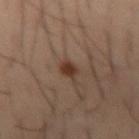Image and clinical context:
A 15 mm close-up tile from a total-body photography series done for melanoma screening. A male patient, aged around 40. From the right forearm. The total-body-photography lesion software estimated a mean CIELAB color near L≈35 a*≈17 b*≈25, roughly 10 lightness units darker than nearby skin, and a normalized lesion–skin contrast near 9.5.